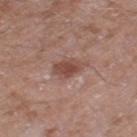This lesion was catalogued during total-body skin photography and was not selected for biopsy.
A region of skin cropped from a whole-body photographic capture, roughly 15 mm wide.
Located on the right thigh.
The tile uses white-light illumination.
The subject is a male aged approximately 60.
Automated tile analysis of the lesion measured a nevus-likeness score of about 45/100 and a lesion-detection confidence of about 100/100.
Longest diameter approximately 4 mm.